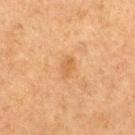lesion_size:
  long_diameter_mm_approx: 2.5
automated_metrics:
  border_irregularity_0_10: 2.5
  color_variation_0_10: 1.5
  peripheral_color_asymmetry: 0.5
image:
  source: total-body photography crop
  field_of_view_mm: 15
patient:
  sex: male
  age_approx: 75
site: right thigh
lighting: cross-polarized